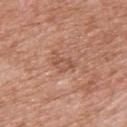biopsy status: total-body-photography surveillance lesion; no biopsy | image: ~15 mm tile from a whole-body skin photo | lesion size: ≈3 mm | subject: male, in their mid-70s | site: the upper back | lighting: white-light illumination.